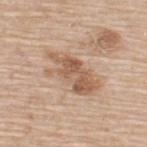{"biopsy_status": "not biopsied; imaged during a skin examination", "site": "upper back", "lesion_size": {"long_diameter_mm_approx": 6.5}, "patient": {"sex": "male", "age_approx": 80}, "image": {"source": "total-body photography crop", "field_of_view_mm": 15}, "lighting": "white-light"}